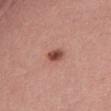Notes:
• follow-up · imaged on a skin check; not biopsied
• diameter · ≈2.5 mm
• subject · female, aged 68–72
• body site · the abdomen
• image source · total-body-photography crop, ~15 mm field of view